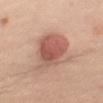follow-up = catalogued during a skin exam; not biopsied
patient = male, aged around 25
anatomic site = the right upper arm
lighting = white-light
size = about 6 mm
image = ~15 mm tile from a whole-body skin photo